A 15 mm close-up tile from a total-body photography series done for melanoma screening. The patient is a male about 70 years old. The lesion is on the head or neck:
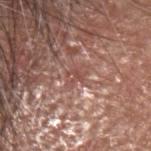<lesion>
<lighting>white-light</lighting>
<lesion_size>
  <long_diameter_mm_approx>2.5</long_diameter_mm_approx>
</lesion_size>
<automated_metrics>
  <nevus_likeness_0_100>0</nevus_likeness_0_100>
  <lesion_detection_confidence_0_100>0</lesion_detection_confidence_0_100>
</automated_metrics>
<diagnosis>
  <histopathology>squamous cell carcinoma in situ</histopathology>
  <malignancy>malignant</malignancy>
  <taxonomic_path>Malignant, Malignant epidermal proliferations, Squamous cell carcinoma in situ</taxonomic_path>
</diagnosis>
</lesion>